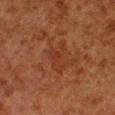Q: Is there a histopathology result?
A: total-body-photography surveillance lesion; no biopsy
Q: What kind of image is this?
A: 15 mm crop, total-body photography
Q: What lighting was used for the tile?
A: cross-polarized illumination
Q: Lesion location?
A: the right lower leg
Q: What are the patient's age and sex?
A: male, in their 80s
Q: What did automated image analysis measure?
A: a lesion area of about 6.5 mm² and a shape eccentricity near 0.85; a within-lesion color-variation index near 1.5/10; a lesion-detection confidence of about 100/100